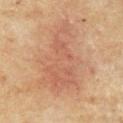The lesion is located on the chest. The patient is a male aged approximately 65. Longest diameter approximately 9.5 mm. Captured under cross-polarized illumination. A 15 mm close-up tile from a total-body photography series done for melanoma screening.